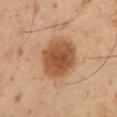Recorded during total-body skin imaging; not selected for excision or biopsy. The lesion is located on the leg. A 15 mm crop from a total-body photograph taken for skin-cancer surveillance. This is a cross-polarized tile. A male patient, approximately 55 years of age. Approximately 5.5 mm at its widest.